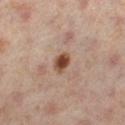follow-up = no biopsy performed (imaged during a skin exam) | tile lighting = cross-polarized illumination | subject = female, aged around 50 | imaging modality = ~15 mm tile from a whole-body skin photo | size = ~2.5 mm (longest diameter) | anatomic site = the left lower leg.